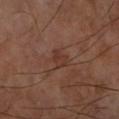Measured at roughly 2.5 mm in maximum diameter.
A male subject, in their 70s.
A region of skin cropped from a whole-body photographic capture, roughly 15 mm wide.
The lesion-visualizer software estimated a lesion area of about 3.5 mm² and two-axis asymmetry of about 0.35. And it measured about 6 CIELAB-L* units darker than the surrounding skin.
The lesion is on the right forearm.
The tile uses cross-polarized illumination.